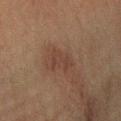The lesion was photographed on a routine skin check and not biopsied; there is no pathology result.
Located on the left forearm.
A male patient approximately 50 years of age.
Longest diameter approximately 3.5 mm.
Cropped from a whole-body photographic skin survey; the tile spans about 15 mm.